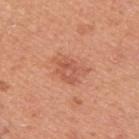Imaged during a routine full-body skin examination; the lesion was not biopsied and no histopathology is available. The total-body-photography lesion software estimated a classifier nevus-likeness of about 40/100. The lesion is located on the upper back. Imaged with white-light lighting. A male patient approximately 45 years of age. Approximately 4 mm at its widest. A close-up tile cropped from a whole-body skin photograph, about 15 mm across.